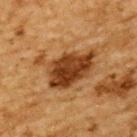Clinical impression:
Recorded during total-body skin imaging; not selected for excision or biopsy.
Image and clinical context:
About 7.5 mm across. Automated image analysis of the tile measured roughly 14 lightness units darker than nearby skin and a normalized lesion–skin contrast near 11.5. And it measured a border-irregularity index near 6/10, internal color variation of about 5 on a 0–10 scale, and peripheral color asymmetry of about 2. And it measured a classifier nevus-likeness of about 45/100 and lesion-presence confidence of about 100/100. A roughly 15 mm field-of-view crop from a total-body skin photograph. Located on the upper back. Imaged with cross-polarized lighting. The subject is a male aged approximately 85 or older.